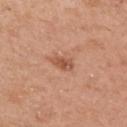Q: How was the tile lit?
A: white-light
Q: Lesion size?
A: ≈2.5 mm
Q: How was this image acquired?
A: ~15 mm tile from a whole-body skin photo
Q: Patient demographics?
A: female, aged around 40
Q: What is the anatomic site?
A: the left upper arm